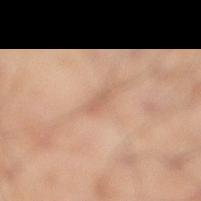Q: Automated lesion metrics?
A: an area of roughly 3.5 mm², an outline eccentricity of about 0.95 (0 = round, 1 = elongated), and a shape-asymmetry score of about 0.3 (0 = symmetric); a lesion color around L≈62 a*≈19 b*≈31 in CIELAB, about 8 CIELAB-L* units darker than the surrounding skin, and a lesion-to-skin contrast of about 5 (normalized; higher = more distinct); a nevus-likeness score of about 0/100 and a detector confidence of about 55 out of 100 that the crop contains a lesion
Q: What is the anatomic site?
A: the right lower leg
Q: How was this image acquired?
A: ~15 mm tile from a whole-body skin photo
Q: Patient demographics?
A: male, in their mid-50s
Q: How was the tile lit?
A: cross-polarized